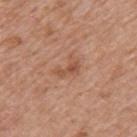Case summary:
– workup: catalogued during a skin exam; not biopsied
– subject: male, in their 60s
– size: about 3 mm
– anatomic site: the back
– tile lighting: white-light illumination
– image: 15 mm crop, total-body photography